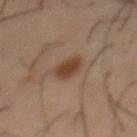biopsy status: total-body-photography surveillance lesion; no biopsy | image source: ~15 mm crop, total-body skin-cancer survey | lesion diameter: ~3 mm (longest diameter) | subject: male, aged 53 to 57 | TBP lesion metrics: border irregularity of about 1.5 on a 0–10 scale and internal color variation of about 2.5 on a 0–10 scale | body site: the chest | tile lighting: cross-polarized.